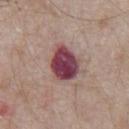Findings:
- notes — no biopsy performed (imaged during a skin exam)